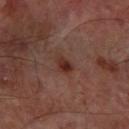Clinical impression: Imaged during a routine full-body skin examination; the lesion was not biopsied and no histopathology is available. Acquisition and patient details: The lesion is located on the left forearm. A male patient in their mid- to late 60s. A region of skin cropped from a whole-body photographic capture, roughly 15 mm wide. Captured under cross-polarized illumination.